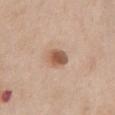Clinical impression: The lesion was photographed on a routine skin check and not biopsied; there is no pathology result. Clinical summary: From the chest. A lesion tile, about 15 mm wide, cut from a 3D total-body photograph. Captured under white-light illumination. Automated tile analysis of the lesion measured a footprint of about 5 mm² and two-axis asymmetry of about 0.15. The software also gave a mean CIELAB color near L≈55 a*≈20 b*≈31 and a normalized lesion–skin contrast near 9. It also reported a border-irregularity rating of about 1.5/10, internal color variation of about 4 on a 0–10 scale, and a peripheral color-asymmetry measure near 1. The software also gave an automated nevus-likeness rating near 95 out of 100 and a lesion-detection confidence of about 100/100. A male patient, aged approximately 60. The recorded lesion diameter is about 2.5 mm.